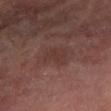Part of a total-body skin-imaging series; this lesion was reviewed on a skin check and was not flagged for biopsy. Measured at roughly 3 mm in maximum diameter. A region of skin cropped from a whole-body photographic capture, roughly 15 mm wide. The total-body-photography lesion software estimated an area of roughly 5.5 mm², an eccentricity of roughly 0.65, and a shape-asymmetry score of about 0.35 (0 = symmetric). It also reported a border-irregularity rating of about 3.5/10, a within-lesion color-variation index near 1.5/10, and radial color variation of about 0.5. The analysis additionally found a nevus-likeness score of about 0/100 and a lesion-detection confidence of about 100/100. This is a cross-polarized tile. The lesion is on the left forearm. The patient is a male aged around 60.